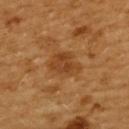Captured during whole-body skin photography for melanoma surveillance; the lesion was not biopsied.
Captured under cross-polarized illumination.
Located on the upper back.
A lesion tile, about 15 mm wide, cut from a 3D total-body photograph.
A female patient, in their mid- to late 50s.
Automated image analysis of the tile measured an area of roughly 7.5 mm², an eccentricity of roughly 0.65, and two-axis asymmetry of about 0.3. The software also gave a lesion–skin lightness drop of about 8 and a lesion-to-skin contrast of about 6.5 (normalized; higher = more distinct). And it measured a border-irregularity index near 2.5/10, a within-lesion color-variation index near 3/10, and peripheral color asymmetry of about 1. The software also gave a nevus-likeness score of about 20/100.